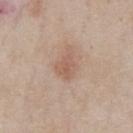biopsy status — total-body-photography surveillance lesion; no biopsy
subject — male, approximately 60 years of age
TBP lesion metrics — a shape-asymmetry score of about 0.4 (0 = symmetric); a lesion color around L≈57 a*≈19 b*≈28 in CIELAB and a lesion-to-skin contrast of about 5.5 (normalized; higher = more distinct); a border-irregularity rating of about 3.5/10 and a color-variation rating of about 2.5/10
imaging modality — ~15 mm crop, total-body skin-cancer survey
lesion size — ~3 mm (longest diameter)
body site — the chest